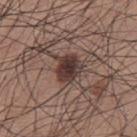| key | value |
|---|---|
| workup | imaged on a skin check; not biopsied |
| anatomic site | the upper back |
| acquisition | ~15 mm crop, total-body skin-cancer survey |
| illumination | white-light |
| subject | male, about 35 years old |